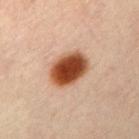Q: Was a biopsy performed?
A: imaged on a skin check; not biopsied
Q: Lesion size?
A: ~4.5 mm (longest diameter)
Q: Where on the body is the lesion?
A: the chest
Q: What lighting was used for the tile?
A: cross-polarized illumination
Q: What kind of image is this?
A: ~15 mm tile from a whole-body skin photo
Q: Patient demographics?
A: female, approximately 30 years of age